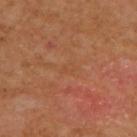Imaged during a routine full-body skin examination; the lesion was not biopsied and no histopathology is available. Imaged with cross-polarized lighting. Approximately 2 mm at its widest. A 15 mm close-up extracted from a 3D total-body photography capture. A male patient aged around 45. From the chest. An algorithmic analysis of the crop reported a lesion color around L≈46 a*≈23 b*≈35 in CIELAB, a lesion–skin lightness drop of about 3, and a normalized lesion–skin contrast near 3. It also reported a border-irregularity rating of about 2/10, a within-lesion color-variation index near 0/10, and radial color variation of about 0.